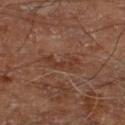A male patient, aged 68–72. This is a cross-polarized tile. The total-body-photography lesion software estimated a footprint of about 7 mm² and two-axis asymmetry of about 0.4. A 15 mm close-up extracted from a 3D total-body photography capture. Measured at roughly 4.5 mm in maximum diameter. On the right lower leg.